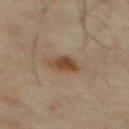Image and clinical context: Captured under cross-polarized illumination. The lesion's longest dimension is about 2.5 mm. On the chest. A male subject, approximately 70 years of age. A 15 mm close-up extracted from a 3D total-body photography capture.